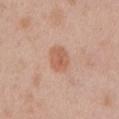Q: Is there a histopathology result?
A: no biopsy performed (imaged during a skin exam)
Q: What are the patient's age and sex?
A: male, aged 48 to 52
Q: Where on the body is the lesion?
A: the left upper arm
Q: What kind of image is this?
A: total-body-photography crop, ~15 mm field of view